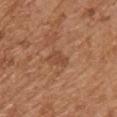Findings:
– biopsy status · no biopsy performed (imaged during a skin exam)
– image · total-body-photography crop, ~15 mm field of view
– body site · the upper back
– subject · male, aged around 70
– lighting · white-light
– image-analysis metrics · a shape-asymmetry score of about 0.3 (0 = symmetric); a mean CIELAB color near L≈47 a*≈23 b*≈33 and a normalized border contrast of about 5.5